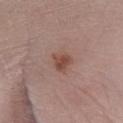Clinical impression:
Captured during whole-body skin photography for melanoma surveillance; the lesion was not biopsied.
Acquisition and patient details:
Measured at roughly 2.5 mm in maximum diameter. Cropped from a whole-body photographic skin survey; the tile spans about 15 mm. A male subject aged around 65. The lesion is located on the left lower leg. Imaged with white-light lighting. The lesion-visualizer software estimated border irregularity of about 3.5 on a 0–10 scale, a within-lesion color-variation index near 2.5/10, and radial color variation of about 1.